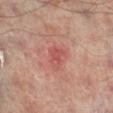| field | value |
|---|---|
| follow-up | imaged on a skin check; not biopsied |
| illumination | cross-polarized |
| image source | ~15 mm tile from a whole-body skin photo |
| body site | the right lower leg |
| subject | male, aged around 65 |